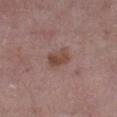Acquisition and patient details: The patient is a male aged approximately 75. A close-up tile cropped from a whole-body skin photograph, about 15 mm across. The lesion is on the right lower leg. The recorded lesion diameter is about 3 mm. This is a white-light tile.